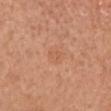  biopsy_status: not biopsied; imaged during a skin examination
  lesion_size:
    long_diameter_mm_approx: 2.0
  image:
    source: total-body photography crop
    field_of_view_mm: 15
  automated_metrics:
    area_mm2_approx: 2.5
    eccentricity: 0.7
    cielab_L: 58
    cielab_a: 25
    cielab_b: 34
    vs_skin_darker_L: 5.0
    vs_skin_contrast_norm: 3.5
    border_irregularity_0_10: 3.0
    color_variation_0_10: 1.0
  patient:
    sex: female
    age_approx: 55
  site: head or neck